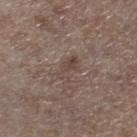The lesion was tiled from a total-body skin photograph and was not biopsied.
A male patient about 70 years old.
Measured at roughly 3 mm in maximum diameter.
The lesion is located on the right lower leg.
Automated tile analysis of the lesion measured a classifier nevus-likeness of about 10/100 and a detector confidence of about 85 out of 100 that the crop contains a lesion.
A close-up tile cropped from a whole-body skin photograph, about 15 mm across.
This is a white-light tile.